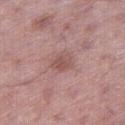biopsy_status: not biopsied; imaged during a skin examination
lesion_size:
  long_diameter_mm_approx: 3.0
image:
  source: total-body photography crop
  field_of_view_mm: 15
site: right thigh
patient:
  sex: male
  age_approx: 50
lighting: white-light